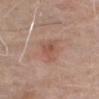Recorded during total-body skin imaging; not selected for excision or biopsy. This image is a 15 mm lesion crop taken from a total-body photograph. Measured at roughly 4 mm in maximum diameter. Located on the arm. The subject is a male aged 73–77.